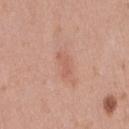Findings:
* follow-up — catalogued during a skin exam; not biopsied
* acquisition — ~15 mm tile from a whole-body skin photo
* patient — female, about 40 years old
* size — ≈3 mm
* lighting — white-light illumination
* body site — the right thigh
* image-analysis metrics — a lesion color around L≈59 a*≈24 b*≈29 in CIELAB and roughly 7 lightness units darker than nearby skin; border irregularity of about 5 on a 0–10 scale, internal color variation of about 0 on a 0–10 scale, and a peripheral color-asymmetry measure near 0; an automated nevus-likeness rating near 10 out of 100 and a detector confidence of about 100 out of 100 that the crop contains a lesion